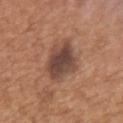A male subject, aged approximately 65. The recorded lesion diameter is about 5 mm. A region of skin cropped from a whole-body photographic capture, roughly 15 mm wide. The total-body-photography lesion software estimated an area of roughly 14 mm², a shape eccentricity near 0.7, and a symmetry-axis asymmetry near 0.25. The analysis additionally found an average lesion color of about L≈44 a*≈19 b*≈25 (CIELAB), about 13 CIELAB-L* units darker than the surrounding skin, and a normalized border contrast of about 10.5. It also reported a border-irregularity index near 3/10, a within-lesion color-variation index near 5/10, and peripheral color asymmetry of about 1.5. The analysis additionally found a classifier nevus-likeness of about 15/100 and a detector confidence of about 100 out of 100 that the crop contains a lesion. The lesion is on the upper back. Imaged with white-light lighting.